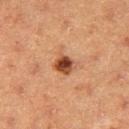Assessment: The lesion was photographed on a routine skin check and not biopsied; there is no pathology result. Image and clinical context: A region of skin cropped from a whole-body photographic capture, roughly 15 mm wide. Measured at roughly 2.5 mm in maximum diameter. The patient is a female aged 38 to 42. On the right thigh.